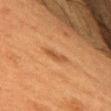Recorded during total-body skin imaging; not selected for excision or biopsy.
Approximately 3 mm at its widest.
A female patient, roughly 50 years of age.
The tile uses cross-polarized illumination.
From the arm.
A region of skin cropped from a whole-body photographic capture, roughly 15 mm wide.